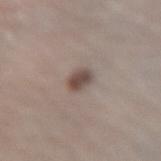<tbp_lesion>
<biopsy_status>not biopsied; imaged during a skin examination</biopsy_status>
</tbp_lesion>